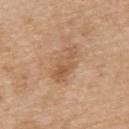Assessment: This lesion was catalogued during total-body skin photography and was not selected for biopsy. Clinical summary: The patient is a male in their 70s. A roughly 15 mm field-of-view crop from a total-body skin photograph. From the upper back. This is a white-light tile.